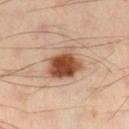workup: imaged on a skin check; not biopsied | automated lesion analysis: border irregularity of about 3 on a 0–10 scale, a color-variation rating of about 6.5/10, and peripheral color asymmetry of about 2; a nevus-likeness score of about 100/100 and a detector confidence of about 100 out of 100 that the crop contains a lesion | site: the leg | image source: ~15 mm crop, total-body skin-cancer survey | subject: male, aged 38 to 42 | lesion size: ≈4.5 mm.